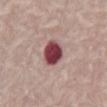Impression:
The lesion was photographed on a routine skin check and not biopsied; there is no pathology result.
Background:
A 15 mm close-up tile from a total-body photography series done for melanoma screening. The lesion is located on the abdomen. A female subject, aged around 65.